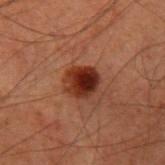| feature | finding |
|---|---|
| follow-up | catalogued during a skin exam; not biopsied |
| TBP lesion metrics | a nevus-likeness score of about 100/100 |
| image | 15 mm crop, total-body photography |
| body site | the left upper arm |
| subject | male, roughly 60 years of age |
| diameter | ~3.5 mm (longest diameter) |